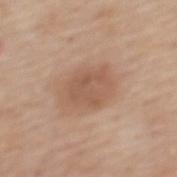Assessment: Captured during whole-body skin photography for melanoma surveillance; the lesion was not biopsied. Background: A close-up tile cropped from a whole-body skin photograph, about 15 mm across. A female patient approximately 65 years of age. The lesion is located on the upper back. Approximately 5 mm at its widest. This is a white-light tile.